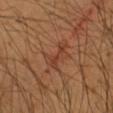Recorded during total-body skin imaging; not selected for excision or biopsy. The tile uses cross-polarized illumination. This image is a 15 mm lesion crop taken from a total-body photograph. On the right upper arm. A male patient in their mid- to late 60s. The lesion's longest dimension is about 3.5 mm. Automated image analysis of the tile measured an area of roughly 6 mm² and an outline eccentricity of about 0.8 (0 = round, 1 = elongated). And it measured a border-irregularity rating of about 6.5/10, a color-variation rating of about 2.5/10, and a peripheral color-asymmetry measure near 1.